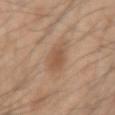Q: Was this lesion biopsied?
A: no biopsy performed (imaged during a skin exam)
Q: What kind of image is this?
A: ~15 mm tile from a whole-body skin photo
Q: How was the tile lit?
A: white-light illumination
Q: What are the patient's age and sex?
A: male, roughly 45 years of age
Q: Lesion size?
A: about 3 mm
Q: What is the anatomic site?
A: the right upper arm